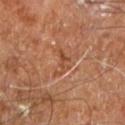Impression:
No biopsy was performed on this lesion — it was imaged during a full skin examination and was not determined to be concerning.
Acquisition and patient details:
An algorithmic analysis of the crop reported a lesion area of about 4 mm², an outline eccentricity of about 0.8 (0 = round, 1 = elongated), and a symmetry-axis asymmetry near 0.7. And it measured a lesion–skin lightness drop of about 7 and a normalized lesion–skin contrast near 5.5. The software also gave a border-irregularity index near 8.5/10, a within-lesion color-variation index near 0/10, and peripheral color asymmetry of about 0. The analysis additionally found a lesion-detection confidence of about 50/100. Longest diameter approximately 3 mm. On the right leg. A 15 mm close-up tile from a total-body photography series done for melanoma screening. Captured under cross-polarized illumination. A male subject, aged around 60.